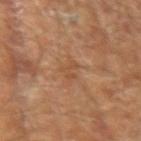Imaged during a routine full-body skin examination; the lesion was not biopsied and no histopathology is available.
The lesion is located on the arm.
A male subject aged around 65.
A 15 mm close-up extracted from a 3D total-body photography capture.